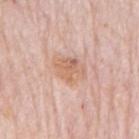Findings:
* notes: imaged on a skin check; not biopsied
* location: the back
* subject: male, aged 78 to 82
* image source: ~15 mm crop, total-body skin-cancer survey